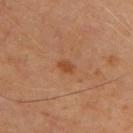Findings:
– notes — catalogued during a skin exam; not biopsied
– anatomic site — the upper back
– size — about 2 mm
– acquisition — total-body-photography crop, ~15 mm field of view
– subject — male, aged approximately 70
– lighting — cross-polarized illumination